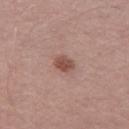A male patient, about 55 years old. About 2.5 mm across. The lesion is on the left thigh. Imaged with white-light lighting. A close-up tile cropped from a whole-body skin photograph, about 15 mm across.